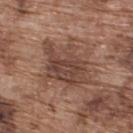workup: total-body-photography surveillance lesion; no biopsy.